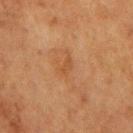workup: imaged on a skin check; not biopsied | lesion diameter: ≈2.5 mm | lighting: cross-polarized | image: ~15 mm crop, total-body skin-cancer survey | anatomic site: the right upper arm | subject: female, in their mid- to late 50s | automated lesion analysis: a lesion color around L≈42 a*≈20 b*≈33 in CIELAB, roughly 5 lightness units darker than nearby skin, and a normalized lesion–skin contrast near 5; a classifier nevus-likeness of about 0/100 and a detector confidence of about 100 out of 100 that the crop contains a lesion.